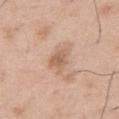Impression:
Part of a total-body skin-imaging series; this lesion was reviewed on a skin check and was not flagged for biopsy.
Clinical summary:
The recorded lesion diameter is about 3 mm. From the leg. This is a white-light tile. A male patient aged approximately 50. A 15 mm close-up extracted from a 3D total-body photography capture.